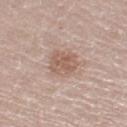<lesion>
  <biopsy_status>not biopsied; imaged during a skin examination</biopsy_status>
  <lesion_size>
    <long_diameter_mm_approx>3.5</long_diameter_mm_approx>
  </lesion_size>
  <patient>
    <sex>female</sex>
    <age_approx>45</age_approx>
  </patient>
  <lighting>white-light</lighting>
  <site>leg</site>
  <automated_metrics>
    <cielab_L>58</cielab_L>
    <cielab_a>18</cielab_a>
    <cielab_b>26</cielab_b>
    <vs_skin_darker_L>9.0</vs_skin_darker_L>
    <vs_skin_contrast_norm>6.5</vs_skin_contrast_norm>
    <border_irregularity_0_10>2.0</border_irregularity_0_10>
    <color_variation_0_10>3.0</color_variation_0_10>
    <peripheral_color_asymmetry>1.0</peripheral_color_asymmetry>
    <nevus_likeness_0_100>40</nevus_likeness_0_100>
    <lesion_detection_confidence_0_100>100</lesion_detection_confidence_0_100>
  </automated_metrics>
  <image>
    <source>total-body photography crop</source>
    <field_of_view_mm>15</field_of_view_mm>
  </image>
</lesion>